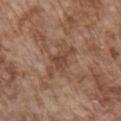Findings:
• biopsy status · total-body-photography surveillance lesion; no biopsy
• subject · male, roughly 75 years of age
• image · 15 mm crop, total-body photography
• body site · the chest